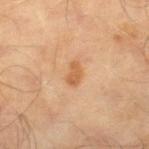biopsy status: no biopsy performed (imaged during a skin exam); image: ~15 mm crop, total-body skin-cancer survey; anatomic site: the leg; illumination: cross-polarized; size: ~2.5 mm (longest diameter); patient: male, approximately 65 years of age.